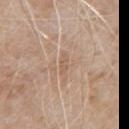<record>
<site>chest</site>
<lighting>white-light</lighting>
<patient>
  <sex>male</sex>
  <age_approx>80</age_approx>
</patient>
<lesion_size>
  <long_diameter_mm_approx>2.5</long_diameter_mm_approx>
</lesion_size>
<image>
  <source>total-body photography crop</source>
  <field_of_view_mm>15</field_of_view_mm>
</image>
<automated_metrics>
  <eccentricity>0.9</eccentricity>
  <shape_asymmetry>0.2</shape_asymmetry>
</automated_metrics>
</record>